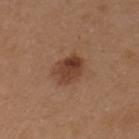image:
  source: total-body photography crop
  field_of_view_mm: 15
patient:
  sex: female
  age_approx: 40
site: right upper arm
lighting: white-light
lesion_size:
  long_diameter_mm_approx: 3.5